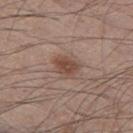Captured during whole-body skin photography for melanoma surveillance; the lesion was not biopsied.
Longest diameter approximately 3 mm.
A roughly 15 mm field-of-view crop from a total-body skin photograph.
A male patient aged 43 to 47.
The lesion is on the left thigh.